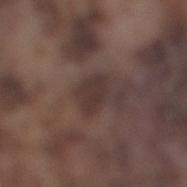Imaged during a routine full-body skin examination; the lesion was not biopsied and no histopathology is available.
The lesion is located on the right lower leg.
The patient is a male approximately 75 years of age.
About 3 mm across.
This is a white-light tile.
A 15 mm close-up extracted from a 3D total-body photography capture.